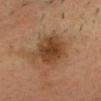Recorded during total-body skin imaging; not selected for excision or biopsy.
The lesion is on the head or neck.
A male patient about 35 years old.
A region of skin cropped from a whole-body photographic capture, roughly 15 mm wide.